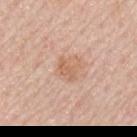lesion diameter: about 3 mm | illumination: white-light illumination | image: total-body-photography crop, ~15 mm field of view | anatomic site: the right upper arm | patient: male, aged 58–62.